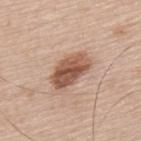follow-up: no biopsy performed (imaged during a skin exam).